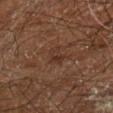A male subject aged around 60.
Automated tile analysis of the lesion measured a lesion area of about 4 mm², an eccentricity of roughly 0.8, and a symmetry-axis asymmetry near 0.35.
Imaged with cross-polarized lighting.
Approximately 3 mm at its widest.
The lesion is located on the right leg.
A 15 mm crop from a total-body photograph taken for skin-cancer surveillance.